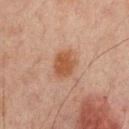<record>
  <biopsy_status>not biopsied; imaged during a skin examination</biopsy_status>
  <image>
    <source>total-body photography crop</source>
    <field_of_view_mm>15</field_of_view_mm>
  </image>
  <patient>
    <sex>male</sex>
    <age_approx>65</age_approx>
  </patient>
  <lighting>cross-polarized</lighting>
  <automated_metrics>
    <area_mm2_approx>7.5</area_mm2_approx>
    <eccentricity>0.65</eccentricity>
    <shape_asymmetry>0.15</shape_asymmetry>
    <color_variation_0_10>2.0</color_variation_0_10>
    <peripheral_color_asymmetry>0.5</peripheral_color_asymmetry>
    <nevus_likeness_0_100>95</nevus_likeness_0_100>
    <lesion_detection_confidence_0_100>100</lesion_detection_confidence_0_100>
  </automated_metrics>
  <site>mid back</site>
</record>